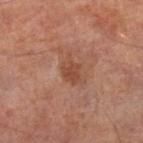biopsy_status: not biopsied; imaged during a skin examination
automated_metrics:
  cielab_L: 44
  cielab_a: 22
  cielab_b: 29
  vs_skin_contrast_norm: 6.5
  border_irregularity_0_10: 3.5
  color_variation_0_10: 2.0
  peripheral_color_asymmetry: 0.5
image:
  source: total-body photography crop
  field_of_view_mm: 15
lesion_size:
  long_diameter_mm_approx: 3.5
lighting: cross-polarized
site: right lower leg
patient:
  sex: male
  age_approx: 65